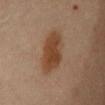No biopsy was performed on this lesion — it was imaged during a full skin examination and was not determined to be concerning.
The tile uses cross-polarized illumination.
A female patient, aged 58–62.
A close-up tile cropped from a whole-body skin photograph, about 15 mm across.
On the chest.
Measured at roughly 6.5 mm in maximum diameter.